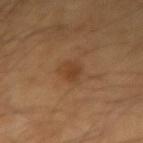{"biopsy_status": "not biopsied; imaged during a skin examination", "site": "left forearm", "lighting": "cross-polarized", "lesion_size": {"long_diameter_mm_approx": 3.0}, "image": {"source": "total-body photography crop", "field_of_view_mm": 15}, "automated_metrics": {"cielab_L": 41, "cielab_a": 20, "cielab_b": 34, "vs_skin_darker_L": 7.0, "vs_skin_contrast_norm": 6.0, "border_irregularity_0_10": 2.0, "color_variation_0_10": 2.0, "peripheral_color_asymmetry": 0.5, "nevus_likeness_0_100": 60}, "patient": {"sex": "male", "age_approx": 65}}